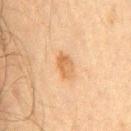Case summary:
– follow-up: imaged on a skin check; not biopsied
– patient: male, approximately 60 years of age
– lighting: cross-polarized
– location: the front of the torso
– diameter: ≈3 mm
– image: total-body-photography crop, ~15 mm field of view